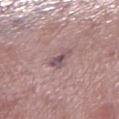• body site: the right lower leg
• subject: male, aged approximately 70
• lesion diameter: ~3 mm (longest diameter)
• image source: ~15 mm tile from a whole-body skin photo